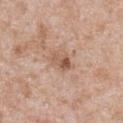Assessment: Recorded during total-body skin imaging; not selected for excision or biopsy. Image and clinical context: On the chest. A male subject roughly 65 years of age. Measured at roughly 3 mm in maximum diameter. Cropped from a whole-body photographic skin survey; the tile spans about 15 mm. This is a white-light tile.